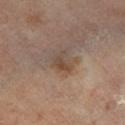This lesion was catalogued during total-body skin photography and was not selected for biopsy.
A male subject, aged 63 to 67.
The tile uses cross-polarized illumination.
Located on the left leg.
The lesion's longest dimension is about 3 mm.
An algorithmic analysis of the crop reported an eccentricity of roughly 0.65 and two-axis asymmetry of about 0.45.
A close-up tile cropped from a whole-body skin photograph, about 15 mm across.